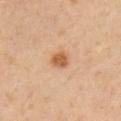| field | value |
|---|---|
| body site | the right upper arm |
| lesion size | ≈2.5 mm |
| patient | male, approximately 45 years of age |
| lighting | cross-polarized |
| acquisition | ~15 mm crop, total-body skin-cancer survey |
| automated lesion analysis | an area of roughly 3.5 mm², a shape eccentricity near 0.6, and a symmetry-axis asymmetry near 0.25; a lesion color around L≈57 a*≈23 b*≈37 in CIELAB and a lesion-to-skin contrast of about 8.5 (normalized; higher = more distinct); a classifier nevus-likeness of about 95/100 and lesion-presence confidence of about 100/100 |